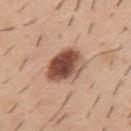The lesion was photographed on a routine skin check and not biopsied; there is no pathology result.
The subject is a male aged 38 to 42.
This is a white-light tile.
The lesion's longest dimension is about 5 mm.
A close-up tile cropped from a whole-body skin photograph, about 15 mm across.
Located on the mid back.